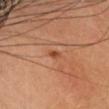Recorded during total-body skin imaging; not selected for excision or biopsy. Measured at roughly 1.5 mm in maximum diameter. Cropped from a whole-body photographic skin survey; the tile spans about 15 mm. The lesion is on the head or neck. A female subject in their 60s. This is a cross-polarized tile.